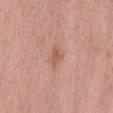Impression: No biopsy was performed on this lesion — it was imaged during a full skin examination and was not determined to be concerning. Background: The total-body-photography lesion software estimated a lesion area of about 3.5 mm², a shape eccentricity near 0.75, and a shape-asymmetry score of about 0.4 (0 = symmetric). It also reported an average lesion color of about L≈57 a*≈24 b*≈29 (CIELAB), a lesion–skin lightness drop of about 8, and a normalized lesion–skin contrast near 6. It also reported a nevus-likeness score of about 5/100 and lesion-presence confidence of about 100/100. The patient is a male aged approximately 70. A 15 mm close-up extracted from a 3D total-body photography capture. From the mid back.